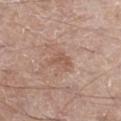Q: Is there a histopathology result?
A: catalogued during a skin exam; not biopsied
Q: How was the tile lit?
A: white-light illumination
Q: Who is the patient?
A: male, in their mid-60s
Q: What is the imaging modality?
A: ~15 mm tile from a whole-body skin photo
Q: Lesion size?
A: ~3 mm (longest diameter)
Q: Lesion location?
A: the left thigh